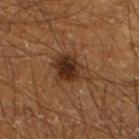Impression:
The lesion was photographed on a routine skin check and not biopsied; there is no pathology result.
Background:
From the leg. Captured under cross-polarized illumination. A male patient, approximately 65 years of age. Cropped from a whole-body photographic skin survey; the tile spans about 15 mm.